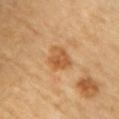<tbp_lesion>
  <biopsy_status>not biopsied; imaged during a skin examination</biopsy_status>
  <image>
    <source>total-body photography crop</source>
    <field_of_view_mm>15</field_of_view_mm>
  </image>
  <automated_metrics>
    <area_mm2_approx>7.5</area_mm2_approx>
    <eccentricity>0.45</eccentricity>
    <shape_asymmetry>0.25</shape_asymmetry>
    <cielab_L>58</cielab_L>
    <cielab_a>22</cielab_a>
    <cielab_b>42</cielab_b>
    <vs_skin_contrast_norm>7.0</vs_skin_contrast_norm>
  </automated_metrics>
  <lesion_size>
    <long_diameter_mm_approx>3.5</long_diameter_mm_approx>
  </lesion_size>
  <site>front of the torso</site>
  <patient>
    <sex>female</sex>
    <age_approx>60</age_approx>
  </patient>
  <lighting>cross-polarized</lighting>
</tbp_lesion>